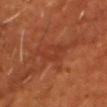This lesion was catalogued during total-body skin photography and was not selected for biopsy.
On the chest.
A 15 mm close-up extracted from a 3D total-body photography capture.
Automated image analysis of the tile measured an eccentricity of roughly 0.85 and a symmetry-axis asymmetry near 0.55. And it measured a lesion color around L≈35 a*≈27 b*≈31 in CIELAB, roughly 5 lightness units darker than nearby skin, and a normalized lesion–skin contrast near 4.5. And it measured a nevus-likeness score of about 0/100 and lesion-presence confidence of about 55/100.
A male patient, aged approximately 55.
Captured under cross-polarized illumination.
The recorded lesion diameter is about 3.5 mm.